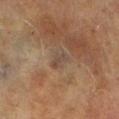| feature | finding |
|---|---|
| workup | catalogued during a skin exam; not biopsied |
| body site | the right lower leg |
| lesion size | about 3 mm |
| acquisition | ~15 mm tile from a whole-body skin photo |
| automated lesion analysis | a lesion area of about 4 mm² and a symmetry-axis asymmetry near 0.35; a border-irregularity index near 3.5/10, a color-variation rating of about 3/10, and peripheral color asymmetry of about 1 |
| subject | male, about 70 years old |